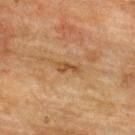The patient is a male aged 73–77. Cropped from a total-body skin-imaging series; the visible field is about 15 mm. Automated tile analysis of the lesion measured about 8 CIELAB-L* units darker than the surrounding skin and a normalized lesion–skin contrast near 7. The analysis additionally found border irregularity of about 4 on a 0–10 scale, a color-variation rating of about 0.5/10, and radial color variation of about 0. And it measured a nevus-likeness score of about 0/100 and lesion-presence confidence of about 100/100. The lesion is on the upper back. This is a cross-polarized tile. The lesion's longest dimension is about 2.5 mm.